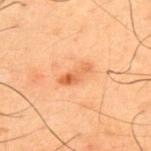notes: imaged on a skin check; not biopsied
subject: male, roughly 50 years of age
location: the upper back
image source: total-body-photography crop, ~15 mm field of view
size: ~4 mm (longest diameter)
tile lighting: cross-polarized
image-analysis metrics: a mean CIELAB color near L≈49 a*≈22 b*≈35, about 8 CIELAB-L* units darker than the surrounding skin, and a lesion-to-skin contrast of about 6.5 (normalized; higher = more distinct); a border-irregularity index near 3/10; an automated nevus-likeness rating near 30 out of 100